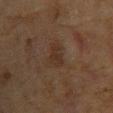{
  "biopsy_status": "not biopsied; imaged during a skin examination",
  "patient": {
    "sex": "female",
    "age_approx": 80
  },
  "image": {
    "source": "total-body photography crop",
    "field_of_view_mm": 15
  },
  "site": "chest",
  "lesion_size": {
    "long_diameter_mm_approx": 3.0
  }
}